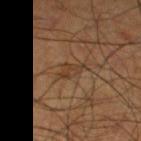Clinical impression:
Imaged during a routine full-body skin examination; the lesion was not biopsied and no histopathology is available.
Image and clinical context:
On the leg. Automated tile analysis of the lesion measured an average lesion color of about L≈37 a*≈17 b*≈30 (CIELAB), about 6 CIELAB-L* units darker than the surrounding skin, and a lesion-to-skin contrast of about 6 (normalized; higher = more distinct). And it measured a detector confidence of about 60 out of 100 that the crop contains a lesion. Imaged with cross-polarized lighting. A male subject aged approximately 60. A roughly 15 mm field-of-view crop from a total-body skin photograph. The recorded lesion diameter is about 3 mm.